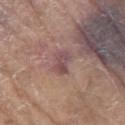Findings:
• notes: no biopsy performed (imaged during a skin exam)
• tile lighting: white-light
• image source: 15 mm crop, total-body photography
• body site: the left upper arm
• subject: male, in their 80s
• automated metrics: roughly 9 lightness units darker than nearby skin and a lesion-to-skin contrast of about 7.5 (normalized; higher = more distinct); a color-variation rating of about 4/10 and a peripheral color-asymmetry measure near 1.5